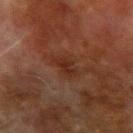workup: imaged on a skin check; not biopsied
acquisition: ~15 mm tile from a whole-body skin photo
site: the left forearm
subject: male, aged 68 to 72
lesion diameter: ~3 mm (longest diameter)
tile lighting: cross-polarized illumination
automated metrics: a footprint of about 4.5 mm², an outline eccentricity of about 0.8 (0 = round, 1 = elongated), and a symmetry-axis asymmetry near 0.25; a border-irregularity rating of about 3.5/10, internal color variation of about 3 on a 0–10 scale, and peripheral color asymmetry of about 1; a classifier nevus-likeness of about 0/100 and a lesion-detection confidence of about 100/100